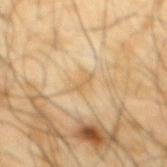Assessment:
This lesion was catalogued during total-body skin photography and was not selected for biopsy.
Image and clinical context:
About 2.5 mm across. The subject is a male aged 63 to 67. Cropped from a whole-body photographic skin survey; the tile spans about 15 mm. From the mid back.